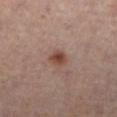Case summary:
- biopsy status · no biopsy performed (imaged during a skin exam)
- body site · the leg
- subject · male, in their 50s
- imaging modality · ~15 mm tile from a whole-body skin photo